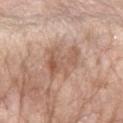follow-up: no biopsy performed (imaged during a skin exam)
body site: the left forearm
diameter: ≈5 mm
image: ~15 mm crop, total-body skin-cancer survey
tile lighting: white-light illumination
subject: female, about 65 years old